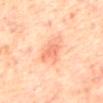Captured during whole-body skin photography for melanoma surveillance; the lesion was not biopsied.
Imaged with cross-polarized lighting.
Approximately 4 mm at its widest.
The subject is a male about 70 years old.
On the mid back.
A 15 mm close-up tile from a total-body photography series done for melanoma screening.